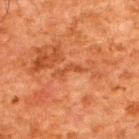Q: Was this lesion biopsied?
A: total-body-photography surveillance lesion; no biopsy
Q: Lesion size?
A: ~3 mm (longest diameter)
Q: What lighting was used for the tile?
A: cross-polarized
Q: Who is the patient?
A: male, aged 58 to 62
Q: Automated lesion metrics?
A: a footprint of about 2.5 mm², an eccentricity of roughly 0.95, and a shape-asymmetry score of about 0.55 (0 = symmetric); border irregularity of about 7 on a 0–10 scale and peripheral color asymmetry of about 0
Q: Where on the body is the lesion?
A: the upper back
Q: What kind of image is this?
A: total-body-photography crop, ~15 mm field of view